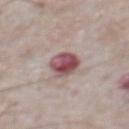– workup: imaged on a skin check; not biopsied
– body site: the abdomen
– subject: male, in their mid-70s
– image-analysis metrics: a lesion color around L≈50 a*≈24 b*≈17 in CIELAB, roughly 16 lightness units darker than nearby skin, and a normalized lesion–skin contrast near 11; a nevus-likeness score of about 5/100 and a lesion-detection confidence of about 100/100
– illumination: white-light
– diameter: ≈4 mm
– image: total-body-photography crop, ~15 mm field of view